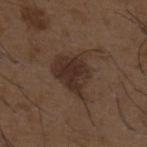notes=catalogued during a skin exam; not biopsied
patient=male, in their 50s
anatomic site=the upper back
image-analysis metrics=an average lesion color of about L≈30 a*≈15 b*≈22 (CIELAB); a classifier nevus-likeness of about 65/100
imaging modality=~15 mm crop, total-body skin-cancer survey
diameter=about 5.5 mm
lighting=white-light